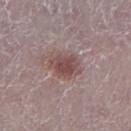Impression: Part of a total-body skin-imaging series; this lesion was reviewed on a skin check and was not flagged for biopsy. Clinical summary: The lesion is on the leg. A close-up tile cropped from a whole-body skin photograph, about 15 mm across. The subject is a female in their mid- to late 60s.